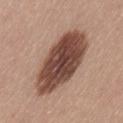Captured during whole-body skin photography for melanoma surveillance; the lesion was not biopsied. A female subject, approximately 55 years of age. A 15 mm close-up extracted from a 3D total-body photography capture. The lesion is on the leg. Measured at roughly 9 mm in maximum diameter.